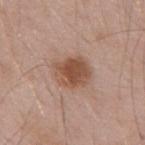<tbp_lesion>
  <biopsy_status>not biopsied; imaged during a skin examination</biopsy_status>
  <image>
    <source>total-body photography crop</source>
    <field_of_view_mm>15</field_of_view_mm>
  </image>
  <patient>
    <sex>male</sex>
    <age_approx>55</age_approx>
  </patient>
  <automated_metrics>
    <color_variation_0_10>4.0</color_variation_0_10>
    <nevus_likeness_0_100>95</nevus_likeness_0_100>
    <lesion_detection_confidence_0_100>100</lesion_detection_confidence_0_100>
  </automated_metrics>
  <site>mid back</site>
  <lesion_size>
    <long_diameter_mm_approx>4.0</long_diameter_mm_approx>
  </lesion_size>
</tbp_lesion>